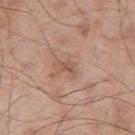Imaged during a routine full-body skin examination; the lesion was not biopsied and no histopathology is available.
The lesion is located on the right upper arm.
A 15 mm crop from a total-body photograph taken for skin-cancer surveillance.
A male patient about 65 years old.